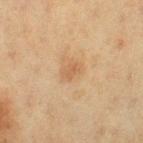Impression:
Imaged during a routine full-body skin examination; the lesion was not biopsied and no histopathology is available.
Background:
The lesion is located on the left thigh. A close-up tile cropped from a whole-body skin photograph, about 15 mm across. A female subject about 50 years old.